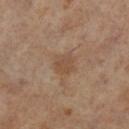Recorded during total-body skin imaging; not selected for excision or biopsy.
On the left lower leg.
A 15 mm close-up tile from a total-body photography series done for melanoma screening.
A female subject roughly 60 years of age.